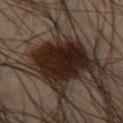biopsy status = catalogued during a skin exam; not biopsied
site = the right thigh
patient = male, aged approximately 50
automated metrics = about 12 CIELAB-L* units darker than the surrounding skin and a normalized border contrast of about 16; a border-irregularity rating of about 2/10, a color-variation rating of about 3.5/10, and radial color variation of about 1
lighting = cross-polarized illumination
image source = ~15 mm crop, total-body skin-cancer survey